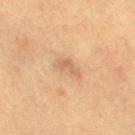A female subject, aged approximately 55. From the leg. This is a cross-polarized tile. Approximately 3 mm at its widest. This image is a 15 mm lesion crop taken from a total-body photograph.